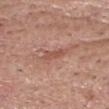| feature | finding |
|---|---|
| size | about 3 mm |
| imaging modality | 15 mm crop, total-body photography |
| location | the head or neck |
| subject | male, in their mid- to late 60s |